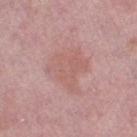No biopsy was performed on this lesion — it was imaged during a full skin examination and was not determined to be concerning.
The subject is a female approximately 60 years of age.
Cropped from a whole-body photographic skin survey; the tile spans about 15 mm.
On the right thigh.
Captured under white-light illumination.
About 5.5 mm across.